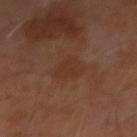Findings:
• biopsy status: total-body-photography surveillance lesion; no biopsy